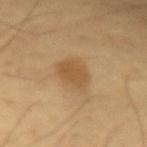| field | value |
|---|---|
| workup | total-body-photography surveillance lesion; no biopsy |
| patient | male, aged approximately 65 |
| tile lighting | cross-polarized |
| image-analysis metrics | a lesion area of about 9 mm² and an outline eccentricity of about 0.6 (0 = round, 1 = elongated); an average lesion color of about L≈54 a*≈17 b*≈38 (CIELAB), about 8 CIELAB-L* units darker than the surrounding skin, and a lesion-to-skin contrast of about 6.5 (normalized; higher = more distinct); a lesion-detection confidence of about 100/100 |
| lesion diameter | about 4 mm |
| image source | total-body-photography crop, ~15 mm field of view |
| site | the left forearm |